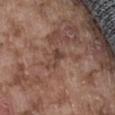– workup: imaged on a skin check; not biopsied
– body site: the abdomen
– tile lighting: white-light illumination
– patient: male, approximately 75 years of age
– lesion diameter: ≈2.5 mm
– image source: total-body-photography crop, ~15 mm field of view
– automated metrics: a footprint of about 2.5 mm² and an eccentricity of roughly 0.85; a classifier nevus-likeness of about 0/100